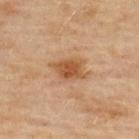Imaged during a routine full-body skin examination; the lesion was not biopsied and no histopathology is available. A 15 mm close-up extracted from a 3D total-body photography capture. A male patient aged around 45. Located on the back.